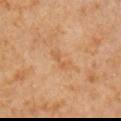Clinical impression: The lesion was photographed on a routine skin check and not biopsied; there is no pathology result. Image and clinical context: A 15 mm close-up tile from a total-body photography series done for melanoma screening. This is a cross-polarized tile. Longest diameter approximately 3 mm. The lesion is on the arm. A male subject, roughly 60 years of age.